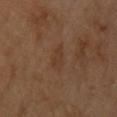Context: A 15 mm close-up extracted from a 3D total-body photography capture. On the upper back. The subject is a male roughly 55 years of age.